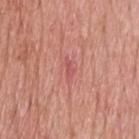This lesion was catalogued during total-body skin photography and was not selected for biopsy.
A roughly 15 mm field-of-view crop from a total-body skin photograph.
The lesion is located on the head or neck.
A male subject approximately 70 years of age.
Longest diameter approximately 2.5 mm.